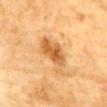Clinical impression:
No biopsy was performed on this lesion — it was imaged during a full skin examination and was not determined to be concerning.
Acquisition and patient details:
Cropped from a total-body skin-imaging series; the visible field is about 15 mm. The subject is a male in their mid- to late 80s or older. The lesion is on the chest.